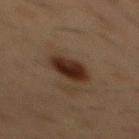A roughly 15 mm field-of-view crop from a total-body skin photograph. This is a cross-polarized tile. From the chest. The patient is a male in their mid- to late 50s.